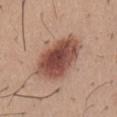The lesion was photographed on a routine skin check and not biopsied; there is no pathology result.